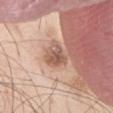Recorded during total-body skin imaging; not selected for excision or biopsy. Automated tile analysis of the lesion measured a mean CIELAB color near L≈56 a*≈20 b*≈28, roughly 11 lightness units darker than nearby skin, and a normalized lesion–skin contrast near 7.5. It also reported radial color variation of about 1. The analysis additionally found an automated nevus-likeness rating near 0 out of 100. Cropped from a whole-body photographic skin survey; the tile spans about 15 mm. Approximately 3.5 mm at its widest. Captured under white-light illumination. A male subject, aged around 70. The lesion is on the abdomen.